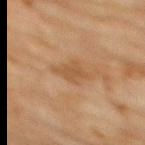follow-up — total-body-photography surveillance lesion; no biopsy | image — ~15 mm tile from a whole-body skin photo | lighting — cross-polarized | diameter — ~4 mm (longest diameter) | subject — female, in their 80s | body site — the upper back | automated lesion analysis — an average lesion color of about L≈45 a*≈17 b*≈33 (CIELAB) and a normalized border contrast of about 6; border irregularity of about 4 on a 0–10 scale, a within-lesion color-variation index near 1.5/10, and radial color variation of about 0.5; a classifier nevus-likeness of about 0/100 and a lesion-detection confidence of about 100/100.